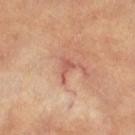Clinical impression:
The lesion was tiled from a total-body skin photograph and was not biopsied.
Image and clinical context:
The patient is aged approximately 60. A 15 mm crop from a total-body photograph taken for skin-cancer surveillance. From the right thigh.